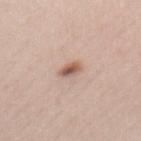* follow-up: no biopsy performed (imaged during a skin exam)